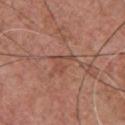Case summary:
- biopsy status · catalogued during a skin exam; not biopsied
- anatomic site · the chest
- tile lighting · white-light illumination
- acquisition · 15 mm crop, total-body photography
- automated lesion analysis · a lesion area of about 4.5 mm²; roughly 7 lightness units darker than nearby skin and a normalized lesion–skin contrast near 5.5; border irregularity of about 7.5 on a 0–10 scale, a color-variation rating of about 0.5/10, and peripheral color asymmetry of about 0; a nevus-likeness score of about 0/100 and lesion-presence confidence of about 70/100
- patient · male, aged 53–57
- size · about 3 mm